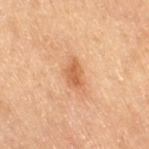Impression:
The lesion was photographed on a routine skin check and not biopsied; there is no pathology result.
Acquisition and patient details:
A female subject, approximately 55 years of age. From the right thigh. Automated tile analysis of the lesion measured a border-irregularity rating of about 3/10 and internal color variation of about 3 on a 0–10 scale. And it measured a nevus-likeness score of about 55/100. About 3.5 mm across. A 15 mm close-up extracted from a 3D total-body photography capture.